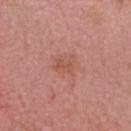Recorded during total-body skin imaging; not selected for excision or biopsy. Measured at roughly 3 mm in maximum diameter. A male subject aged approximately 75. Automated image analysis of the tile measured roughly 6 lightness units darker than nearby skin. The software also gave internal color variation of about 2.5 on a 0–10 scale and radial color variation of about 1. And it measured a nevus-likeness score of about 0/100 and lesion-presence confidence of about 100/100. Cropped from a whole-body photographic skin survey; the tile spans about 15 mm. The tile uses white-light illumination. The lesion is located on the head or neck.